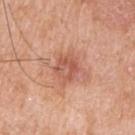<lesion>
  <biopsy_status>not biopsied; imaged during a skin examination</biopsy_status>
  <site>left upper arm</site>
  <automated_metrics>
    <cielab_L>57</cielab_L>
    <cielab_a>27</cielab_a>
    <cielab_b>31</cielab_b>
    <vs_skin_darker_L>10.0</vs_skin_darker_L>
    <vs_skin_contrast_norm>6.5</vs_skin_contrast_norm>
  </automated_metrics>
  <lesion_size>
    <long_diameter_mm_approx>3.5</long_diameter_mm_approx>
  </lesion_size>
  <image>
    <source>total-body photography crop</source>
    <field_of_view_mm>15</field_of_view_mm>
  </image>
  <patient>
    <sex>male</sex>
    <age_approx>60</age_approx>
  </patient>
  <lighting>white-light</lighting>
</lesion>